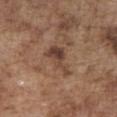Recorded during total-body skin imaging; not selected for excision or biopsy. Longest diameter approximately 4.5 mm. Cropped from a whole-body photographic skin survey; the tile spans about 15 mm. Captured under white-light illumination. The lesion is on the chest. A male patient, in their mid- to late 70s.